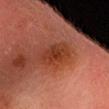Imaged during a routine full-body skin examination; the lesion was not biopsied and no histopathology is available. The lesion is located on the head or neck. A close-up tile cropped from a whole-body skin photograph, about 15 mm across. A male subject, in their mid- to late 30s.